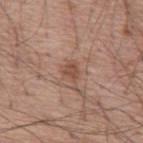The lesion was photographed on a routine skin check and not biopsied; there is no pathology result. The lesion is on the mid back. The subject is a male roughly 55 years of age. Imaged with white-light lighting. A close-up tile cropped from a whole-body skin photograph, about 15 mm across. The lesion's longest dimension is about 2.5 mm.